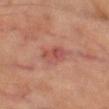workup = no biopsy performed (imaged during a skin exam) | image source = 15 mm crop, total-body photography | TBP lesion metrics = an area of roughly 5 mm²; a mean CIELAB color near L≈49 a*≈27 b*≈27, about 8 CIELAB-L* units darker than the surrounding skin, and a normalized border contrast of about 6; a detector confidence of about 100 out of 100 that the crop contains a lesion | tile lighting = cross-polarized | anatomic site = the left thigh | diameter = ~3 mm (longest diameter) | patient = male, aged approximately 70.